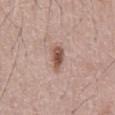follow-up: no biopsy performed (imaged during a skin exam)
tile lighting: white-light
imaging modality: ~15 mm tile from a whole-body skin photo
patient: male, aged 53 to 57